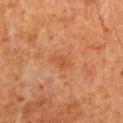{"automated_metrics": {"area_mm2_approx": 4.0, "cielab_L": 41, "cielab_a": 22, "cielab_b": 32, "vs_skin_darker_L": 5.0, "vs_skin_contrast_norm": 5.0, "color_variation_0_10": 1.0, "peripheral_color_asymmetry": 0.5, "nevus_likeness_0_100": 0, "lesion_detection_confidence_0_100": 100}, "lighting": "cross-polarized", "site": "abdomen", "image": {"source": "total-body photography crop", "field_of_view_mm": 15}, "lesion_size": {"long_diameter_mm_approx": 2.5}, "patient": {"sex": "male", "age_approx": 80}}